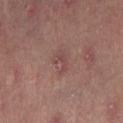Part of a total-body skin-imaging series; this lesion was reviewed on a skin check and was not flagged for biopsy.
The patient is a female aged 48 to 52.
A close-up tile cropped from a whole-body skin photograph, about 15 mm across.
The lesion is on the right thigh.